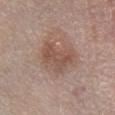Q: Is there a histopathology result?
A: catalogued during a skin exam; not biopsied